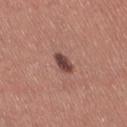Impression:
This lesion was catalogued during total-body skin photography and was not selected for biopsy.
Image and clinical context:
A 15 mm close-up extracted from a 3D total-body photography capture. This is a white-light tile. A female patient, approximately 35 years of age. Automated tile analysis of the lesion measured an area of roughly 4 mm² and a shape eccentricity near 0.85. It also reported border irregularity of about 1.5 on a 0–10 scale, a within-lesion color-variation index near 3/10, and peripheral color asymmetry of about 1. About 3 mm across. The lesion is located on the left thigh.